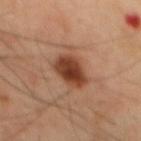Findings:
* location: the mid back
* lighting: cross-polarized
* subject: male, aged 43–47
* lesion size: about 4.5 mm
* acquisition: total-body-photography crop, ~15 mm field of view
* TBP lesion metrics: a mean CIELAB color near L≈39 a*≈23 b*≈30, roughly 14 lightness units darker than nearby skin, and a lesion-to-skin contrast of about 11.5 (normalized; higher = more distinct)